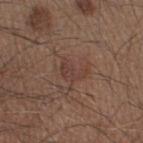Case summary:
– workup — catalogued during a skin exam; not biopsied
– tile lighting — white-light illumination
– image — 15 mm crop, total-body photography
– location — the right thigh
– automated metrics — a lesion color around L≈39 a*≈18 b*≈23 in CIELAB and about 6 CIELAB-L* units darker than the surrounding skin
– patient — male, aged around 45
– size — ~3 mm (longest diameter)